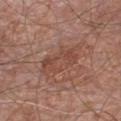Impression:
The lesion was tiled from a total-body skin photograph and was not biopsied.